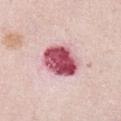notes: total-body-photography surveillance lesion; no biopsy | lighting: white-light | image source: 15 mm crop, total-body photography | TBP lesion metrics: an area of roughly 15 mm², an eccentricity of roughly 0.65, and a symmetry-axis asymmetry near 0.1; a border-irregularity rating of about 1/10, a within-lesion color-variation index near 8/10, and peripheral color asymmetry of about 3; an automated nevus-likeness rating near 0 out of 100 and lesion-presence confidence of about 100/100 | lesion diameter: ~5 mm (longest diameter) | subject: female, aged around 65 | anatomic site: the abdomen.